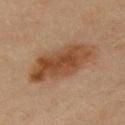Findings:
* follow-up — catalogued during a skin exam; not biopsied
* tile lighting — cross-polarized illumination
* subject — female, in their mid-40s
* size — ~8 mm (longest diameter)
* image — ~15 mm tile from a whole-body skin photo
* body site — the left upper arm